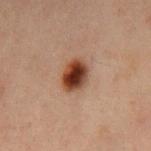TBP lesion metrics — a border-irregularity rating of about 1.5/10, internal color variation of about 7.5 on a 0–10 scale, and radial color variation of about 2 | image source — ~15 mm tile from a whole-body skin photo | diameter — ≈3.5 mm | body site — the mid back | illumination — cross-polarized | patient — male, approximately 60 years of age.